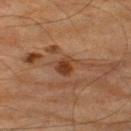Context: Imaged with cross-polarized lighting. The lesion is on the left thigh. Approximately 3.5 mm at its widest. A male subject, roughly 85 years of age or older. Automated tile analysis of the lesion measured roughly 8 lightness units darker than nearby skin. A region of skin cropped from a whole-body photographic capture, roughly 15 mm wide.